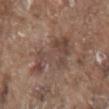Impression:
Captured during whole-body skin photography for melanoma surveillance; the lesion was not biopsied.
Acquisition and patient details:
Imaged with white-light lighting. A close-up tile cropped from a whole-body skin photograph, about 15 mm across. The recorded lesion diameter is about 6.5 mm. A male patient, aged approximately 80. From the mid back.